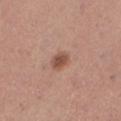Q: Was this lesion biopsied?
A: imaged on a skin check; not biopsied